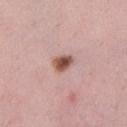Assessment: Part of a total-body skin-imaging series; this lesion was reviewed on a skin check and was not flagged for biopsy. Clinical summary: A 15 mm crop from a total-body photograph taken for skin-cancer surveillance. From the left lower leg. A female subject, approximately 35 years of age.